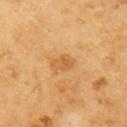No biopsy was performed on this lesion — it was imaged during a full skin examination and was not determined to be concerning. On the right upper arm. A 15 mm close-up extracted from a 3D total-body photography capture. A male patient, approximately 60 years of age.